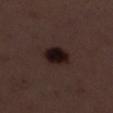Q: Was this lesion biopsied?
A: imaged on a skin check; not biopsied
Q: Illumination type?
A: white-light
Q: What is the imaging modality?
A: 15 mm crop, total-body photography
Q: Lesion location?
A: the left lower leg
Q: Patient demographics?
A: female, approximately 50 years of age
Q: How large is the lesion?
A: ~3.5 mm (longest diameter)
Q: Automated lesion metrics?
A: a shape eccentricity near 0.6 and two-axis asymmetry of about 0.15; a lesion color around L≈16 a*≈13 b*≈14 in CIELAB, about 11 CIELAB-L* units darker than the surrounding skin, and a normalized lesion–skin contrast near 14.5; a border-irregularity index near 1/10, a within-lesion color-variation index near 4.5/10, and peripheral color asymmetry of about 1